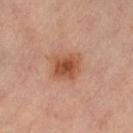follow-up — catalogued during a skin exam; not biopsied
site — the left lower leg
illumination — cross-polarized illumination
subject — female, about 50 years old
image — 15 mm crop, total-body photography
lesion size — about 3.5 mm
automated metrics — a classifier nevus-likeness of about 95/100 and a detector confidence of about 100 out of 100 that the crop contains a lesion